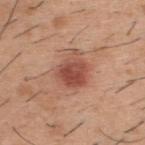biopsy_status: not biopsied; imaged during a skin examination
patient:
  sex: male
  age_approx: 40
image:
  source: total-body photography crop
  field_of_view_mm: 15
lesion_size:
  long_diameter_mm_approx: 4.0
site: back
lighting: white-light